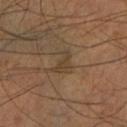{
  "biopsy_status": "not biopsied; imaged during a skin examination",
  "site": "left lower leg",
  "image": {
    "source": "total-body photography crop",
    "field_of_view_mm": 15
  },
  "lesion_size": {
    "long_diameter_mm_approx": 3.0
  },
  "lighting": "cross-polarized",
  "automated_metrics": {
    "area_mm2_approx": 4.0,
    "eccentricity": 0.8,
    "shape_asymmetry": 0.5,
    "border_irregularity_0_10": 5.0,
    "color_variation_0_10": 1.5,
    "peripheral_color_asymmetry": 0.5
  },
  "patient": {
    "sex": "male",
    "age_approx": 55
  }
}